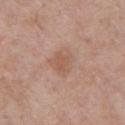Assessment:
Captured during whole-body skin photography for melanoma surveillance; the lesion was not biopsied.
Context:
An algorithmic analysis of the crop reported an area of roughly 8.5 mm², a shape eccentricity near 0.5, and a shape-asymmetry score of about 0.3 (0 = symmetric). It also reported border irregularity of about 3 on a 0–10 scale, a within-lesion color-variation index near 3/10, and peripheral color asymmetry of about 1. A male patient, approximately 55 years of age. The lesion is on the chest. Longest diameter approximately 3 mm. Imaged with white-light lighting. Cropped from a whole-body photographic skin survey; the tile spans about 15 mm.